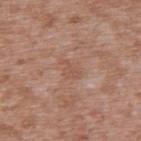Case summary:
- workup: total-body-photography surveillance lesion; no biopsy
- location: the upper back
- image: total-body-photography crop, ~15 mm field of view
- size: about 2.5 mm
- patient: male, in their mid-40s